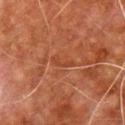| feature | finding |
|---|---|
| notes | no biopsy performed (imaged during a skin exam) |
| patient | male, aged approximately 80 |
| diameter | ~3 mm (longest diameter) |
| image source | 15 mm crop, total-body photography |
| illumination | cross-polarized |
| anatomic site | the front of the torso |
| automated metrics | an outline eccentricity of about 0.9 (0 = round, 1 = elongated) and two-axis asymmetry of about 0.45; a color-variation rating of about 0/10 and a peripheral color-asymmetry measure near 0; a nevus-likeness score of about 0/100 and a lesion-detection confidence of about 80/100 |